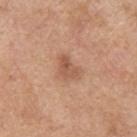Imaged during a routine full-body skin examination; the lesion was not biopsied and no histopathology is available.
A region of skin cropped from a whole-body photographic capture, roughly 15 mm wide.
The tile uses white-light illumination.
A male patient aged around 60.
The lesion is on the upper back.
Longest diameter approximately 3.5 mm.